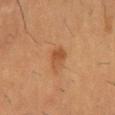Impression:
No biopsy was performed on this lesion — it was imaged during a full skin examination and was not determined to be concerning.
Context:
The lesion is on the abdomen. An algorithmic analysis of the crop reported an outline eccentricity of about 0.75 (0 = round, 1 = elongated). It also reported a border-irregularity index near 2/10, a within-lesion color-variation index near 3.5/10, and radial color variation of about 1. A region of skin cropped from a whole-body photographic capture, roughly 15 mm wide. A male subject, aged around 55. The recorded lesion diameter is about 3 mm.